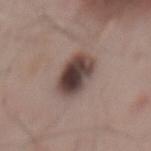Clinical impression:
No biopsy was performed on this lesion — it was imaged during a full skin examination and was not determined to be concerning.
Acquisition and patient details:
Imaged with white-light lighting. About 5 mm across. A male patient, aged 53 to 57. A 15 mm close-up tile from a total-body photography series done for melanoma screening. On the mid back.